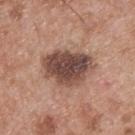Impression:
Recorded during total-body skin imaging; not selected for excision or biopsy.
Acquisition and patient details:
Approximately 5.5 mm at its widest. A male patient, aged 53–57. On the upper back. A close-up tile cropped from a whole-body skin photograph, about 15 mm across.